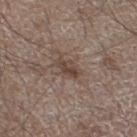  biopsy_status: not biopsied; imaged during a skin examination
  site: left lower leg
  lesion_size:
    long_diameter_mm_approx: 3.0
  patient:
    sex: male
    age_approx: 60
  image:
    source: total-body photography crop
    field_of_view_mm: 15
  automated_metrics:
    border_irregularity_0_10: 2.5
    color_variation_0_10: 3.0
    peripheral_color_asymmetry: 1.0